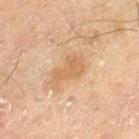workup: no biopsy performed (imaged during a skin exam) | automated lesion analysis: a footprint of about 5.5 mm², an outline eccentricity of about 0.85 (0 = round, 1 = elongated), and a symmetry-axis asymmetry near 0.5; a mean CIELAB color near L≈51 a*≈17 b*≈32 | tile lighting: cross-polarized illumination | imaging modality: ~15 mm tile from a whole-body skin photo | site: the left thigh | subject: male, in their 70s.